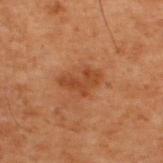Notes:
– lesion size · about 4.5 mm
– image · ~15 mm crop, total-body skin-cancer survey
– image-analysis metrics · a lesion area of about 7.5 mm², a shape eccentricity near 0.8, and a symmetry-axis asymmetry near 0.4; an average lesion color of about L≈34 a*≈21 b*≈30 (CIELAB), a lesion–skin lightness drop of about 7, and a normalized lesion–skin contrast near 6.5; a border-irregularity rating of about 4.5/10, internal color variation of about 2.5 on a 0–10 scale, and peripheral color asymmetry of about 1
– lighting · cross-polarized
– site · the upper back
– subject · male, roughly 70 years of age